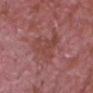This lesion was catalogued during total-body skin photography and was not selected for biopsy. The tile uses white-light illumination. Cropped from a whole-body photographic skin survey; the tile spans about 15 mm. A male subject about 65 years old. On the chest. Approximately 6 mm at its widest.